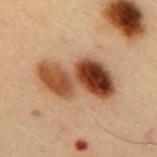<record>
<biopsy_status>not biopsied; imaged during a skin examination</biopsy_status>
<patient>
  <sex>male</sex>
  <age_approx>55</age_approx>
</patient>
<automated_metrics>
  <area_mm2_approx>23.0</area_mm2_approx>
  <eccentricity>0.85</eccentricity>
  <shape_asymmetry>0.35</shape_asymmetry>
  <nevus_likeness_0_100>100</nevus_likeness_0_100>
  <lesion_detection_confidence_0_100>100</lesion_detection_confidence_0_100>
</automated_metrics>
<image>
  <source>total-body photography crop</source>
  <field_of_view_mm>15</field_of_view_mm>
</image>
<site>mid back</site>
<lighting>cross-polarized</lighting>
</record>